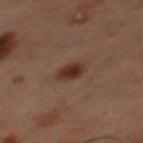Q: Was a biopsy performed?
A: total-body-photography surveillance lesion; no biopsy
Q: What is the imaging modality?
A: total-body-photography crop, ~15 mm field of view
Q: How large is the lesion?
A: about 2.5 mm
Q: What is the anatomic site?
A: the back
Q: Patient demographics?
A: male, aged approximately 60
Q: How was the tile lit?
A: cross-polarized illumination